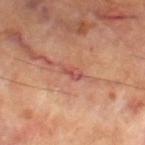The subject is a male in their 70s. Cropped from a total-body skin-imaging series; the visible field is about 15 mm. The lesion is on the left thigh.